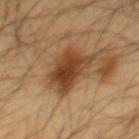Clinical summary: A male subject, aged around 60. A 15 mm close-up extracted from a 3D total-body photography capture. On the abdomen.